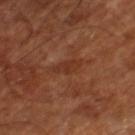<case>
  <biopsy_status>not biopsied; imaged during a skin examination</biopsy_status>
  <lighting>cross-polarized</lighting>
  <image>
    <source>total-body photography crop</source>
    <field_of_view_mm>15</field_of_view_mm>
  </image>
  <site>right lower leg</site>
  <patient>
    <age_approx>65</age_approx>
  </patient>
  <automated_metrics>
    <vs_skin_darker_L>5.0</vs_skin_darker_L>
    <vs_skin_contrast_norm>5.0</vs_skin_contrast_norm>
    <nevus_likeness_0_100>0</nevus_likeness_0_100>
    <lesion_detection_confidence_0_100>95</lesion_detection_confidence_0_100>
  </automated_metrics>
</case>